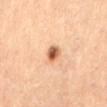<lesion>
<biopsy_status>not biopsied; imaged during a skin examination</biopsy_status>
<image>
  <source>total-body photography crop</source>
  <field_of_view_mm>15</field_of_view_mm>
</image>
<automated_metrics>
  <area_mm2_approx>4.0</area_mm2_approx>
  <eccentricity>0.65</eccentricity>
  <cielab_L>57</cielab_L>
  <cielab_a>23</cielab_a>
  <cielab_b>35</cielab_b>
  <vs_skin_contrast_norm>10.0</vs_skin_contrast_norm>
  <border_irregularity_0_10>2.0</border_irregularity_0_10>
  <color_variation_0_10>6.0</color_variation_0_10>
  <nevus_likeness_0_100>100</nevus_likeness_0_100>
  <lesion_detection_confidence_0_100>100</lesion_detection_confidence_0_100>
</automated_metrics>
<patient>
  <sex>female</sex>
  <age_approx>60</age_approx>
</patient>
<site>lower back</site>
<lighting>cross-polarized</lighting>
<lesion_size>
  <long_diameter_mm_approx>2.5</long_diameter_mm_approx>
</lesion_size>
</lesion>